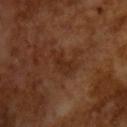Case summary:
• biopsy status: no biopsy performed (imaged during a skin exam)
• image: total-body-photography crop, ~15 mm field of view
• patient: male, aged approximately 65
• automated lesion analysis: a mean CIELAB color near L≈27 a*≈21 b*≈28 and a lesion-to-skin contrast of about 6.5 (normalized; higher = more distinct); a border-irregularity index near 4.5/10, a color-variation rating of about 1.5/10, and peripheral color asymmetry of about 0.5; a classifier nevus-likeness of about 0/100 and a detector confidence of about 100 out of 100 that the crop contains a lesion